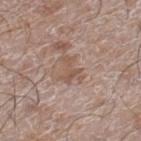Q: Is there a histopathology result?
A: no biopsy performed (imaged during a skin exam)
Q: What are the patient's age and sex?
A: male, aged 58–62
Q: How was this image acquired?
A: 15 mm crop, total-body photography
Q: Illumination type?
A: white-light
Q: How large is the lesion?
A: about 3 mm
Q: Where on the body is the lesion?
A: the leg